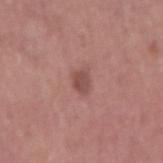Q: Is there a histopathology result?
A: total-body-photography surveillance lesion; no biopsy
Q: Automated lesion metrics?
A: an area of roughly 4 mm², an outline eccentricity of about 0.7 (0 = round, 1 = elongated), and two-axis asymmetry of about 0.3; border irregularity of about 2.5 on a 0–10 scale, a within-lesion color-variation index near 1.5/10, and a peripheral color-asymmetry measure near 0.5; a lesion-detection confidence of about 100/100
Q: Illumination type?
A: white-light
Q: Lesion location?
A: the right upper arm
Q: How was this image acquired?
A: total-body-photography crop, ~15 mm field of view
Q: Patient demographics?
A: male, aged around 60